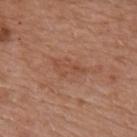<tbp_lesion>
<biopsy_status>not biopsied; imaged during a skin examination</biopsy_status>
<lesion_size>
  <long_diameter_mm_approx>3.5</long_diameter_mm_approx>
</lesion_size>
<site>upper back</site>
<automated_metrics>
  <area_mm2_approx>5.0</area_mm2_approx>
  <shape_asymmetry>0.4</shape_asymmetry>
  <border_irregularity_0_10>4.5</border_irregularity_0_10>
  <color_variation_0_10>2.0</color_variation_0_10>
  <nevus_likeness_0_100>0</nevus_likeness_0_100>
  <lesion_detection_confidence_0_100>100</lesion_detection_confidence_0_100>
</automated_metrics>
<patient>
  <sex>female</sex>
  <age_approx>60</age_approx>
</patient>
<image>
  <source>total-body photography crop</source>
  <field_of_view_mm>15</field_of_view_mm>
</image>
<lighting>white-light</lighting>
</tbp_lesion>